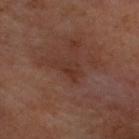  lesion_size:
    long_diameter_mm_approx: 3.5
  image:
    source: total-body photography crop
    field_of_view_mm: 15
  site: head or neck
  automated_metrics:
    eccentricity: 0.85
    cielab_L: 31
    cielab_a: 20
    cielab_b: 25
    color_variation_0_10: 3.0
    nevus_likeness_0_100: 0
    lesion_detection_confidence_0_100: 100
  patient:
    sex: male
    age_approx: 60